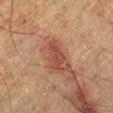follow-up: no biopsy performed (imaged during a skin exam)